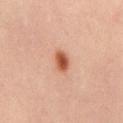Captured during whole-body skin photography for melanoma surveillance; the lesion was not biopsied. Located on the abdomen. A male subject aged around 65. Measured at roughly 3 mm in maximum diameter. Automated image analysis of the tile measured a footprint of about 5.5 mm², a shape eccentricity near 0.7, and two-axis asymmetry of about 0.2. The software also gave a mean CIELAB color near L≈55 a*≈25 b*≈35, about 13 CIELAB-L* units darker than the surrounding skin, and a normalized border contrast of about 9.5. It also reported a border-irregularity rating of about 2/10, a within-lesion color-variation index near 5.5/10, and radial color variation of about 1.5. This image is a 15 mm lesion crop taken from a total-body photograph. This is a cross-polarized tile.